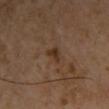No biopsy was performed on this lesion — it was imaged during a full skin examination and was not determined to be concerning. A close-up tile cropped from a whole-body skin photograph, about 15 mm across. The patient is a male about 50 years old. Imaged with cross-polarized lighting. The lesion is located on the chest. Approximately 3 mm at its widest.